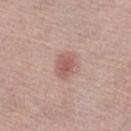notes=imaged on a skin check; not biopsied | site=the left lower leg | patient=female, aged 63 to 67 | acquisition=total-body-photography crop, ~15 mm field of view.